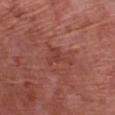{"biopsy_status": "not biopsied; imaged during a skin examination", "site": "chest", "image": {"source": "total-body photography crop", "field_of_view_mm": 15}, "patient": {"sex": "male", "age_approx": 80}}